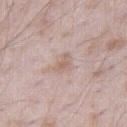Findings:
* biopsy status · imaged on a skin check; not biopsied
* subject · male, aged around 45
* image · ~15 mm tile from a whole-body skin photo
* site · the right thigh
* tile lighting · white-light illumination
* lesion diameter · ≈2.5 mm